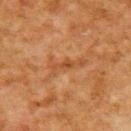Image and clinical context:
This is a cross-polarized tile. Located on the upper back. A male subject about 80 years old. A roughly 15 mm field-of-view crop from a total-body skin photograph.